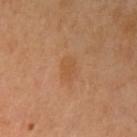{
  "biopsy_status": "not biopsied; imaged during a skin examination",
  "automated_metrics": {
    "cielab_L": 54,
    "cielab_a": 22,
    "cielab_b": 38,
    "vs_skin_darker_L": 5.0,
    "vs_skin_contrast_norm": 5.0,
    "nevus_likeness_0_100": 0,
    "lesion_detection_confidence_0_100": 100
  },
  "image": {
    "source": "total-body photography crop",
    "field_of_view_mm": 15
  },
  "lighting": "cross-polarized",
  "patient": {
    "sex": "female",
    "age_approx": 60
  },
  "site": "left upper arm"
}